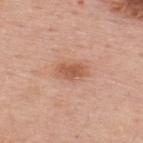This lesion was catalogued during total-body skin photography and was not selected for biopsy.